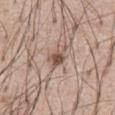Notes:
• notes — catalogued during a skin exam; not biopsied
• tile lighting — white-light
• patient — male, aged approximately 55
• anatomic site — the chest
• size — ~3.5 mm (longest diameter)
• acquisition — 15 mm crop, total-body photography